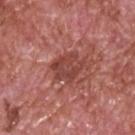Imaged during a routine full-body skin examination; the lesion was not biopsied and no histopathology is available.